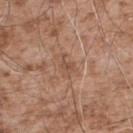Part of a total-body skin-imaging series; this lesion was reviewed on a skin check and was not flagged for biopsy. A male subject aged around 55. A lesion tile, about 15 mm wide, cut from a 3D total-body photograph. Imaged with white-light lighting. Measured at roughly 3 mm in maximum diameter. From the upper back. The lesion-visualizer software estimated a footprint of about 4 mm² and a symmetry-axis asymmetry near 0.4. The analysis additionally found an average lesion color of about L≈51 a*≈19 b*≈30 (CIELAB), about 7 CIELAB-L* units darker than the surrounding skin, and a lesion-to-skin contrast of about 5.5 (normalized; higher = more distinct). The analysis additionally found a color-variation rating of about 2.5/10 and a peripheral color-asymmetry measure near 1. The software also gave an automated nevus-likeness rating near 0 out of 100 and lesion-presence confidence of about 100/100.